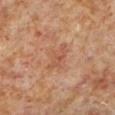Q: Is there a histopathology result?
A: no biopsy performed (imaged during a skin exam)
Q: What is the imaging modality?
A: total-body-photography crop, ~15 mm field of view
Q: What is the anatomic site?
A: the left lower leg
Q: How large is the lesion?
A: ≈3.5 mm
Q: How was the tile lit?
A: cross-polarized
Q: Who is the patient?
A: male, in their 60s